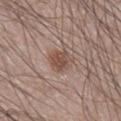Q: Was a biopsy performed?
A: no biopsy performed (imaged during a skin exam)
Q: Patient demographics?
A: male, approximately 60 years of age
Q: Automated lesion metrics?
A: a footprint of about 6 mm², an eccentricity of roughly 0.6, and a symmetry-axis asymmetry near 0.35; a mean CIELAB color near L≈49 a*≈18 b*≈24 and a normalized border contrast of about 7.5; a nevus-likeness score of about 80/100 and a detector confidence of about 100 out of 100 that the crop contains a lesion
Q: What lighting was used for the tile?
A: white-light
Q: What is the imaging modality?
A: ~15 mm tile from a whole-body skin photo
Q: How large is the lesion?
A: ~3.5 mm (longest diameter)
Q: What is the anatomic site?
A: the right lower leg